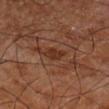{
  "lesion_size": {
    "long_diameter_mm_approx": 2.5
  },
  "site": "left thigh",
  "patient": {
    "sex": "male",
    "age_approx": 80
  },
  "image": {
    "source": "total-body photography crop",
    "field_of_view_mm": 15
  }
}